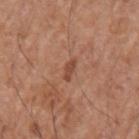Findings:
• notes: imaged on a skin check; not biopsied
• image: 15 mm crop, total-body photography
• patient: male, in their mid- to late 60s
• site: the left upper arm